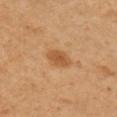  biopsy_status: not biopsied; imaged during a skin examination
  lesion_size:
    long_diameter_mm_approx: 3.5
  lighting: cross-polarized
  site: right upper arm
  patient:
    sex: female
    age_approx: 50
  image:
    source: total-body photography crop
    field_of_view_mm: 15